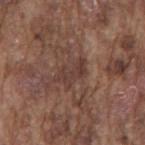Clinical impression:
The lesion was photographed on a routine skin check and not biopsied; there is no pathology result.
Image and clinical context:
From the mid back. An algorithmic analysis of the crop reported an area of roughly 5.5 mm², a shape eccentricity near 0.85, and a shape-asymmetry score of about 0.4 (0 = symmetric). And it measured border irregularity of about 6 on a 0–10 scale, a within-lesion color-variation index near 3/10, and peripheral color asymmetry of about 1. The software also gave a classifier nevus-likeness of about 10/100 and a lesion-detection confidence of about 70/100. The lesion's longest dimension is about 3.5 mm. The patient is a male roughly 75 years of age. Imaged with white-light lighting. This image is a 15 mm lesion crop taken from a total-body photograph.